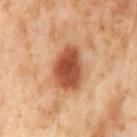The lesion was photographed on a routine skin check and not biopsied; there is no pathology result.
The lesion is on the left thigh.
A 15 mm close-up extracted from a 3D total-body photography capture.
This is a cross-polarized tile.
The subject is a female in their mid- to late 50s.
The lesion-visualizer software estimated an area of roughly 15 mm², a shape eccentricity near 0.8, and a shape-asymmetry score of about 0.15 (0 = symmetric). The software also gave a within-lesion color-variation index near 5/10 and peripheral color asymmetry of about 1. The analysis additionally found a nevus-likeness score of about 100/100.
The recorded lesion diameter is about 5.5 mm.